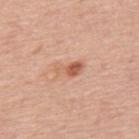follow-up = no biopsy performed (imaged during a skin exam)
site = the mid back
subject = male, aged 73–77
imaging modality = ~15 mm crop, total-body skin-cancer survey